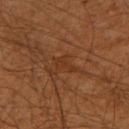| feature | finding |
|---|---|
| notes | catalogued during a skin exam; not biopsied |
| image | 15 mm crop, total-body photography |
| patient | male, roughly 60 years of age |
| body site | the arm |
| lesion size | about 3 mm |